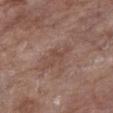Recorded during total-body skin imaging; not selected for excision or biopsy. The tile uses white-light illumination. A close-up tile cropped from a whole-body skin photograph, about 15 mm across. The lesion is on the chest. A female patient, in their mid- to late 70s. The lesion's longest dimension is about 4 mm.